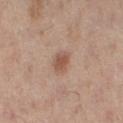{"biopsy_status": "not biopsied; imaged during a skin examination", "automated_metrics": {"area_mm2_approx": 4.5, "eccentricity": 0.6, "shape_asymmetry": 0.15, "cielab_L": 43, "cielab_a": 16, "cielab_b": 23, "vs_skin_darker_L": 8.0, "vs_skin_contrast_norm": 7.0, "nevus_likeness_0_100": 85, "lesion_detection_confidence_0_100": 100}, "site": "left leg", "lesion_size": {"long_diameter_mm_approx": 2.5}, "patient": {"sex": "female", "age_approx": 55}, "image": {"source": "total-body photography crop", "field_of_view_mm": 15}}